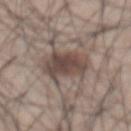No biopsy was performed on this lesion — it was imaged during a full skin examination and was not determined to be concerning.